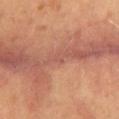The lesion was tiled from a total-body skin photograph and was not biopsied. Measured at roughly 1 mm in maximum diameter. The patient is a female about 60 years old. Cropped from a total-body skin-imaging series; the visible field is about 15 mm. On the mid back. Imaged with cross-polarized lighting.